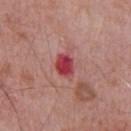Imaged during a routine full-body skin examination; the lesion was not biopsied and no histopathology is available. A male subject, about 70 years old. Approximately 3 mm at its widest. Located on the chest. This image is a 15 mm lesion crop taken from a total-body photograph.